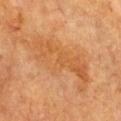No biopsy was performed on this lesion — it was imaged during a full skin examination and was not determined to be concerning. Located on the front of the torso. This is a cross-polarized tile. An algorithmic analysis of the crop reported an area of roughly 22 mm², a shape eccentricity near 0.95, and a shape-asymmetry score of about 0.45 (0 = symmetric). The software also gave a mean CIELAB color near L≈50 a*≈22 b*≈38, a lesion–skin lightness drop of about 6, and a normalized border contrast of about 5.5. And it measured a classifier nevus-likeness of about 0/100. About 9 mm across. A roughly 15 mm field-of-view crop from a total-body skin photograph. A male subject, in their mid- to late 80s or older.